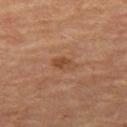The lesion was tiled from a total-body skin photograph and was not biopsied.
The subject is a female aged around 60.
An algorithmic analysis of the crop reported border irregularity of about 2 on a 0–10 scale, a within-lesion color-variation index near 2.5/10, and peripheral color asymmetry of about 1. The software also gave lesion-presence confidence of about 100/100.
On the left thigh.
A lesion tile, about 15 mm wide, cut from a 3D total-body photograph.
Captured under cross-polarized illumination.